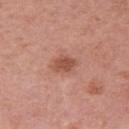Case summary:
- acquisition — 15 mm crop, total-body photography
- site — the right upper arm
- illumination — white-light illumination
- subject — female, about 50 years old